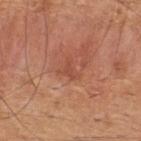A region of skin cropped from a whole-body photographic capture, roughly 15 mm wide.
A male subject, aged around 45.
From the upper back.
The lesion's longest dimension is about 2.5 mm.
The tile uses white-light illumination.